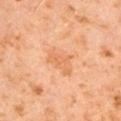notes = no biopsy performed (imaged during a skin exam) | automated metrics = a border-irregularity index near 4/10 and a within-lesion color-variation index near 2.5/10 | tile lighting = cross-polarized | location = the left upper arm | image source = ~15 mm crop, total-body skin-cancer survey | patient = male, about 60 years old | lesion size = ≈4 mm.